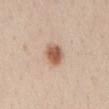The recorded lesion diameter is about 3 mm. The lesion is located on the chest. A female subject aged approximately 45. Cropped from a total-body skin-imaging series; the visible field is about 15 mm. The lesion-visualizer software estimated a lesion-detection confidence of about 100/100.